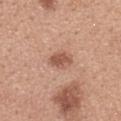Imaged during a routine full-body skin examination; the lesion was not biopsied and no histopathology is available. A female patient roughly 30 years of age. This image is a 15 mm lesion crop taken from a total-body photograph. On the upper back.